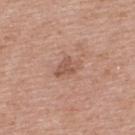Part of a total-body skin-imaging series; this lesion was reviewed on a skin check and was not flagged for biopsy. A male patient, roughly 60 years of age. On the upper back. The recorded lesion diameter is about 3 mm. A lesion tile, about 15 mm wide, cut from a 3D total-body photograph. This is a white-light tile.